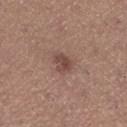Notes:
* biopsy status · imaged on a skin check; not biopsied
* illumination · white-light
* lesion size · ~2.5 mm (longest diameter)
* acquisition · ~15 mm tile from a whole-body skin photo
* patient · female, in their mid-20s
* location · the left lower leg